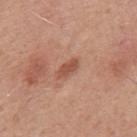{
  "biopsy_status": "not biopsied; imaged during a skin examination",
  "site": "mid back",
  "patient": {
    "sex": "male",
    "age_approx": 50
  },
  "image": {
    "source": "total-body photography crop",
    "field_of_view_mm": 15
  }
}